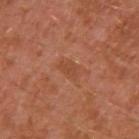lesion diameter = ≈2.5 mm; automated lesion analysis = an automated nevus-likeness rating near 0 out of 100; tile lighting = cross-polarized illumination; patient = male, roughly 30 years of age; image source = ~15 mm tile from a whole-body skin photo; location = the left upper arm.